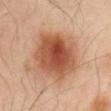follow-up: imaged on a skin check; not biopsied | patient: male, aged around 65 | automated metrics: a footprint of about 29 mm² and a shape eccentricity near 0.6 | lighting: cross-polarized illumination | location: the back | image source: ~15 mm tile from a whole-body skin photo.